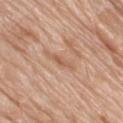Q: Is there a histopathology result?
A: catalogued during a skin exam; not biopsied
Q: What is the lesion's diameter?
A: ≈3 mm
Q: How was the tile lit?
A: white-light
Q: Who is the patient?
A: female, aged around 75
Q: What is the anatomic site?
A: the right thigh
Q: Automated lesion metrics?
A: a color-variation rating of about 0/10 and a peripheral color-asymmetry measure near 0; a nevus-likeness score of about 0/100 and lesion-presence confidence of about 85/100
Q: What kind of image is this?
A: ~15 mm crop, total-body skin-cancer survey